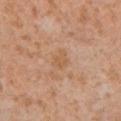Imaged during a routine full-body skin examination; the lesion was not biopsied and no histopathology is available. Captured under cross-polarized illumination. Automated image analysis of the tile measured an area of roughly 4 mm². The software also gave a lesion color around L≈55 a*≈19 b*≈34 in CIELAB, roughly 5 lightness units darker than nearby skin, and a normalized border contrast of about 4.5. And it measured lesion-presence confidence of about 100/100. On the left lower leg. Longest diameter approximately 2.5 mm. The patient is a male aged 28 to 32. A region of skin cropped from a whole-body photographic capture, roughly 15 mm wide.